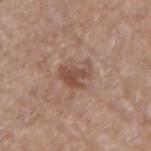The lesion was photographed on a routine skin check and not biopsied; there is no pathology result.
From the left forearm.
A male subject, in their mid-60s.
This image is a 15 mm lesion crop taken from a total-body photograph.
This is a white-light tile.
The total-body-photography lesion software estimated an average lesion color of about L≈49 a*≈19 b*≈27 (CIELAB), about 9 CIELAB-L* units darker than the surrounding skin, and a lesion-to-skin contrast of about 7 (normalized; higher = more distinct). The software also gave a nevus-likeness score of about 35/100 and lesion-presence confidence of about 100/100.